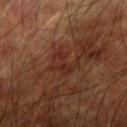Clinical impression:
The lesion was photographed on a routine skin check and not biopsied; there is no pathology result.
Acquisition and patient details:
A male patient approximately 60 years of age. Cropped from a total-body skin-imaging series; the visible field is about 15 mm. Located on the left upper arm.